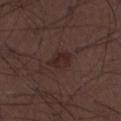Acquisition and patient details:
This image is a 15 mm lesion crop taken from a total-body photograph. The lesion is located on the left lower leg. A male patient, aged 48 to 52. Longest diameter approximately 3 mm. Captured under white-light illumination.